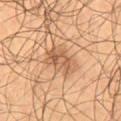biopsy_status: not biopsied; imaged during a skin examination
image:
  source: total-body photography crop
  field_of_view_mm: 15
lesion_size:
  long_diameter_mm_approx: 4.0
lighting: cross-polarized
patient:
  sex: male
  age_approx: 55
site: right thigh
automated_metrics:
  area_mm2_approx: 8.5
  eccentricity: 0.75
  shape_asymmetry: 0.3
  cielab_L: 53
  cielab_a: 20
  cielab_b: 33
  nevus_likeness_0_100: 25
  lesion_detection_confidence_0_100: 100